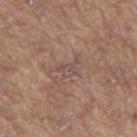follow-up=total-body-photography surveillance lesion; no biopsy
illumination=white-light
body site=the mid back
subject=male, aged approximately 65
image-analysis metrics=an area of roughly 3.5 mm² and two-axis asymmetry of about 0.65; border irregularity of about 7 on a 0–10 scale, a color-variation rating of about 2/10, and peripheral color asymmetry of about 1
image source=15 mm crop, total-body photography